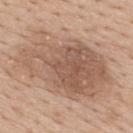Case summary:
– follow-up · imaged on a skin check; not biopsied
– size · about 11.5 mm
– anatomic site · the mid back
– subject · male, in their 60s
– image · ~15 mm crop, total-body skin-cancer survey
– image-analysis metrics · a lesion color around L≈57 a*≈18 b*≈28 in CIELAB and a lesion–skin lightness drop of about 11; a border-irregularity index near 4/10 and a color-variation rating of about 5.5/10; lesion-presence confidence of about 100/100